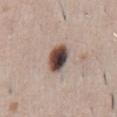Part of a total-body skin-imaging series; this lesion was reviewed on a skin check and was not flagged for biopsy. A male patient aged 48–52. Measured at roughly 4 mm in maximum diameter. This is a white-light tile. A 15 mm close-up tile from a total-body photography series done for melanoma screening. From the front of the torso.